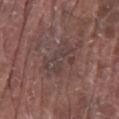Clinical impression: Recorded during total-body skin imaging; not selected for excision or biopsy. Clinical summary: Measured at roughly 5 mm in maximum diameter. The patient is a male in their 80s. Automated image analysis of the tile measured a lesion area of about 12 mm², a shape eccentricity near 0.85, and a shape-asymmetry score of about 0.3 (0 = symmetric). It also reported a normalized border contrast of about 5. The analysis additionally found an automated nevus-likeness rating near 0 out of 100. This is a white-light tile. A 15 mm close-up tile from a total-body photography series done for melanoma screening. From the mid back.